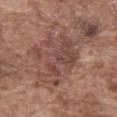  biopsy_status: not biopsied; imaged during a skin examination
  image:
    source: total-body photography crop
    field_of_view_mm: 15
  site: front of the torso
  automated_metrics:
    cielab_L: 46
    cielab_a: 21
    cielab_b: 24
    vs_skin_darker_L: 8.0
    nevus_likeness_0_100: 0
    lesion_detection_confidence_0_100: 85
  patient:
    sex: male
    age_approx: 75
  lighting: white-light